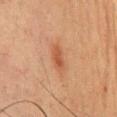From the chest.
Cropped from a total-body skin-imaging series; the visible field is about 15 mm.
Longest diameter approximately 3 mm.
A male patient, aged 48 to 52.
Captured under cross-polarized illumination.